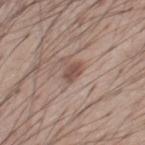follow-up: catalogued during a skin exam; not biopsied
subject: male, aged 53 to 57
illumination: white-light illumination
image: ~15 mm tile from a whole-body skin photo
location: the chest
diameter: ~2.5 mm (longest diameter)